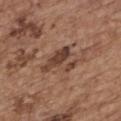Q: Is there a histopathology result?
A: total-body-photography surveillance lesion; no biopsy
Q: What kind of image is this?
A: total-body-photography crop, ~15 mm field of view
Q: Lesion location?
A: the upper back
Q: Who is the patient?
A: female, roughly 65 years of age